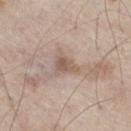workup=catalogued during a skin exam; not biopsied | lesion diameter=about 3 mm | image source=~15 mm tile from a whole-body skin photo | tile lighting=white-light illumination | body site=the right thigh | patient=male, approximately 60 years of age | automated lesion analysis=a lesion area of about 4 mm², an eccentricity of roughly 0.75, and two-axis asymmetry of about 0.35; a lesion color around L≈56 a*≈16 b*≈25 in CIELAB and about 10 CIELAB-L* units darker than the surrounding skin; a border-irregularity index near 3/10, a color-variation rating of about 4/10, and peripheral color asymmetry of about 1.5; an automated nevus-likeness rating near 0 out of 100 and a detector confidence of about 100 out of 100 that the crop contains a lesion.